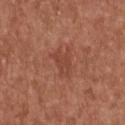This lesion was catalogued during total-body skin photography and was not selected for biopsy. An algorithmic analysis of the crop reported a lesion area of about 5.5 mm². The subject is a female about 65 years old. Captured under white-light illumination. The lesion's longest dimension is about 3.5 mm. A 15 mm close-up extracted from a 3D total-body photography capture. The lesion is on the chest.